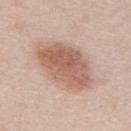workup — total-body-photography surveillance lesion; no biopsy | anatomic site — the upper back | patient — female, about 40 years old | illumination — white-light illumination | TBP lesion metrics — a mean CIELAB color near L≈60 a*≈20 b*≈28 and about 12 CIELAB-L* units darker than the surrounding skin | image — 15 mm crop, total-body photography.